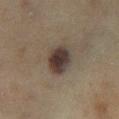Q: Patient demographics?
A: female, approximately 60 years of age
Q: What kind of image is this?
A: total-body-photography crop, ~15 mm field of view
Q: What is the anatomic site?
A: the left lower leg